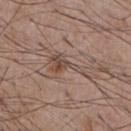| key | value |
|---|---|
| biopsy status | catalogued during a skin exam; not biopsied |
| illumination | white-light illumination |
| anatomic site | the chest |
| subject | male, about 55 years old |
| automated lesion analysis | an average lesion color of about L≈48 a*≈16 b*≈24 (CIELAB) and a normalized lesion–skin contrast near 6.5; internal color variation of about 5.5 on a 0–10 scale and peripheral color asymmetry of about 2; an automated nevus-likeness rating near 5 out of 100 |
| imaging modality | ~15 mm crop, total-body skin-cancer survey |